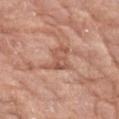<tbp_lesion>
  <biopsy_status>not biopsied; imaged during a skin examination</biopsy_status>
  <lighting>white-light</lighting>
  <site>left forearm</site>
  <image>
    <source>total-body photography crop</source>
    <field_of_view_mm>15</field_of_view_mm>
  </image>
  <patient>
    <sex>female</sex>
    <age_approx>75</age_approx>
  </patient>
  <lesion_size>
    <long_diameter_mm_approx>4.0</long_diameter_mm_approx>
  </lesion_size>
  <automated_metrics>
    <shape_asymmetry>0.4</shape_asymmetry>
    <vs_skin_darker_L>9.0</vs_skin_darker_L>
    <vs_skin_contrast_norm>6.0</vs_skin_contrast_norm>
    <border_irregularity_0_10>5.0</border_irregularity_0_10>
    <color_variation_0_10>3.5</color_variation_0_10>
    <peripheral_color_asymmetry>1.0</peripheral_color_asymmetry>
  </automated_metrics>
</tbp_lesion>